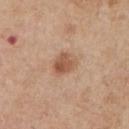workup = no biopsy performed (imaged during a skin exam)
subject = male, about 65 years old
acquisition = ~15 mm crop, total-body skin-cancer survey
location = the chest